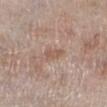- biopsy status: no biopsy performed (imaged during a skin exam)
- image-analysis metrics: a lesion area of about 3 mm², an eccentricity of roughly 0.85, and a symmetry-axis asymmetry near 0.25
- lesion diameter: about 2.5 mm
- illumination: white-light illumination
- imaging modality: ~15 mm tile from a whole-body skin photo
- location: the right lower leg
- subject: male, approximately 60 years of age